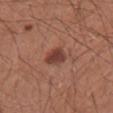Assessment: No biopsy was performed on this lesion — it was imaged during a full skin examination and was not determined to be concerning. Context: The lesion's longest dimension is about 3 mm. The total-body-photography lesion software estimated a mean CIELAB color near L≈41 a*≈24 b*≈26, roughly 11 lightness units darker than nearby skin, and a normalized border contrast of about 9. It also reported a border-irregularity index near 2/10 and radial color variation of about 1. A male subject about 30 years old. A 15 mm close-up tile from a total-body photography series done for melanoma screening. Imaged with white-light lighting. The lesion is on the right forearm.